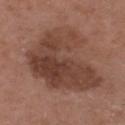A male subject, in their 50s. Cropped from a total-body skin-imaging series; the visible field is about 15 mm. Imaged with white-light lighting. About 9 mm across. Automated image analysis of the tile measured a nevus-likeness score of about 5/100 and a detector confidence of about 100 out of 100 that the crop contains a lesion. The lesion is located on the head or neck.